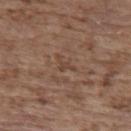Case summary:
- biopsy status · no biopsy performed (imaged during a skin exam)
- subject · female, in their mid-60s
- TBP lesion metrics · internal color variation of about 0 on a 0–10 scale and peripheral color asymmetry of about 0; an automated nevus-likeness rating near 0 out of 100 and lesion-presence confidence of about 55/100
- body site · the upper back
- lighting · white-light illumination
- image · ~15 mm crop, total-body skin-cancer survey